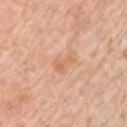follow-up = catalogued during a skin exam; not biopsied
image source = 15 mm crop, total-body photography
site = the left upper arm
patient = male, about 75 years old
diameter = about 2.5 mm